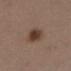Captured during whole-body skin photography for melanoma surveillance; the lesion was not biopsied. A 15 mm close-up extracted from a 3D total-body photography capture. A female subject, about 40 years old. Imaged with white-light lighting. On the leg.